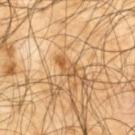Captured during whole-body skin photography for melanoma surveillance; the lesion was not biopsied.
Located on the upper back.
Cropped from a whole-body photographic skin survey; the tile spans about 15 mm.
About 3 mm across.
Captured under cross-polarized illumination.
A male patient, aged 63–67.